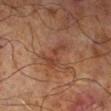Notes:
* biopsy status: no biopsy performed (imaged during a skin exam)
* body site: the right lower leg
* automated lesion analysis: a lesion color around L≈34 a*≈19 b*≈25 in CIELAB and a lesion-to-skin contrast of about 5.5 (normalized; higher = more distinct); internal color variation of about 2.5 on a 0–10 scale and a peripheral color-asymmetry measure near 0.5; an automated nevus-likeness rating near 0 out of 100
* lesion size: about 4.5 mm
* image: 15 mm crop, total-body photography
* patient: male, aged around 70